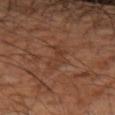Impression: Imaged during a routine full-body skin examination; the lesion was not biopsied and no histopathology is available. Background: Captured under cross-polarized illumination. Located on the right forearm. A male patient, about 55 years old. Cropped from a total-body skin-imaging series; the visible field is about 15 mm. An algorithmic analysis of the crop reported an average lesion color of about L≈38 a*≈21 b*≈29 (CIELAB), about 5 CIELAB-L* units darker than the surrounding skin, and a normalized lesion–skin contrast near 4.5. The analysis additionally found a border-irregularity index near 5/10 and a peripheral color-asymmetry measure near 1.